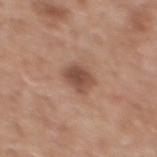Impression: This lesion was catalogued during total-body skin photography and was not selected for biopsy. Acquisition and patient details: About 3 mm across. The tile uses white-light illumination. A male subject aged 58 to 62. Located on the back. A 15 mm close-up extracted from a 3D total-body photography capture.